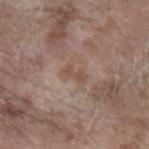Assessment: This lesion was catalogued during total-body skin photography and was not selected for biopsy. Context: The subject is a female roughly 75 years of age. The tile uses white-light illumination. Cropped from a total-body skin-imaging series; the visible field is about 15 mm. Automated image analysis of the tile measured a shape eccentricity near 0.9 and a shape-asymmetry score of about 0.3 (0 = symmetric). It also reported a nevus-likeness score of about 0/100 and lesion-presence confidence of about 100/100. Located on the left forearm.